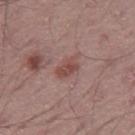notes = imaged on a skin check; not biopsied | site = the right thigh | illumination = white-light | imaging modality = 15 mm crop, total-body photography | subject = male, about 50 years old | diameter = ~3 mm (longest diameter).